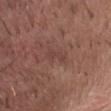Recorded during total-body skin imaging; not selected for excision or biopsy.
Located on the abdomen.
Measured at roughly 2.5 mm in maximum diameter.
A 15 mm crop from a total-body photograph taken for skin-cancer surveillance.
This is a white-light tile.
The subject is a female about 45 years old.